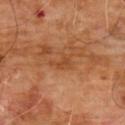Clinical impression: The lesion was photographed on a routine skin check and not biopsied; there is no pathology result. Context: An algorithmic analysis of the crop reported an automated nevus-likeness rating near 0 out of 100 and a detector confidence of about 100 out of 100 that the crop contains a lesion. A male subject, approximately 60 years of age. From the chest. A lesion tile, about 15 mm wide, cut from a 3D total-body photograph.